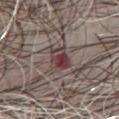  biopsy_status: not biopsied; imaged during a skin examination
  lighting: white-light
  lesion_size:
    long_diameter_mm_approx: 3.0
  patient:
    sex: male
    age_approx: 55
  site: chest
  image:
    source: total-body photography crop
    field_of_view_mm: 15